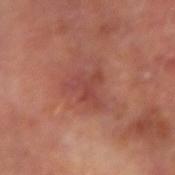Background:
This image is a 15 mm lesion crop taken from a total-body photograph. The recorded lesion diameter is about 4 mm. The lesion is on the left forearm. The patient is a male aged 63 to 67. Imaged with cross-polarized lighting.